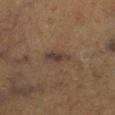Clinical impression:
The lesion was photographed on a routine skin check and not biopsied; there is no pathology result.
Background:
The lesion is on the right lower leg. Imaged with cross-polarized lighting. Cropped from a whole-body photographic skin survey; the tile spans about 15 mm. A male subject, aged around 75. The lesion's longest dimension is about 3.5 mm. An algorithmic analysis of the crop reported a footprint of about 5 mm², a shape eccentricity near 0.9, and a shape-asymmetry score of about 0.3 (0 = symmetric).